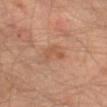This lesion was catalogued during total-body skin photography and was not selected for biopsy. The lesion is on the left thigh. A roughly 15 mm field-of-view crop from a total-body skin photograph. The subject is a male aged around 65. Captured under cross-polarized illumination.